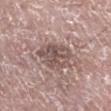The lesion was tiled from a total-body skin photograph and was not biopsied.
On the right lower leg.
A roughly 15 mm field-of-view crop from a total-body skin photograph.
The patient is a male in their mid-70s.